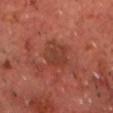Recorded during total-body skin imaging; not selected for excision or biopsy.
A male patient, aged 68–72.
On the front of the torso.
Cropped from a total-body skin-imaging series; the visible field is about 15 mm.
An algorithmic analysis of the crop reported an area of roughly 8 mm² and two-axis asymmetry of about 0.15. The analysis additionally found a lesion–skin lightness drop of about 6 and a normalized lesion–skin contrast near 5.5. The software also gave internal color variation of about 2.5 on a 0–10 scale. The analysis additionally found a nevus-likeness score of about 0/100 and a lesion-detection confidence of about 100/100.